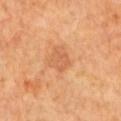Imaged during a routine full-body skin examination; the lesion was not biopsied and no histopathology is available.
A region of skin cropped from a whole-body photographic capture, roughly 15 mm wide.
Automated tile analysis of the lesion measured roughly 7 lightness units darker than nearby skin and a normalized lesion–skin contrast near 4.5. The analysis additionally found a border-irregularity rating of about 3/10, a color-variation rating of about 2/10, and a peripheral color-asymmetry measure near 1. And it measured a classifier nevus-likeness of about 0/100 and a detector confidence of about 100 out of 100 that the crop contains a lesion.
From the left upper arm.
About 2.5 mm across.
A male patient roughly 65 years of age.